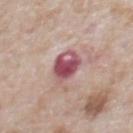Impression:
The lesion was photographed on a routine skin check and not biopsied; there is no pathology result.
Background:
A close-up tile cropped from a whole-body skin photograph, about 15 mm across. The lesion is located on the abdomen. The tile uses white-light illumination. A male subject, aged 58 to 62.